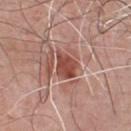- workup: catalogued during a skin exam; not biopsied
- subject: male, aged 58–62
- location: the chest
- imaging modality: 15 mm crop, total-body photography